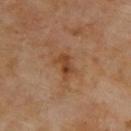The lesion was tiled from a total-body skin photograph and was not biopsied. This image is a 15 mm lesion crop taken from a total-body photograph. The recorded lesion diameter is about 3 mm. The lesion is on the upper back. The tile uses cross-polarized illumination. The subject is a male aged 68 to 72.